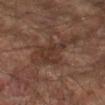Imaged during a routine full-body skin examination; the lesion was not biopsied and no histopathology is available. A roughly 15 mm field-of-view crop from a total-body skin photograph. About 7 mm across. Imaged with cross-polarized lighting. An algorithmic analysis of the crop reported about 7 CIELAB-L* units darker than the surrounding skin and a normalized lesion–skin contrast near 6.5. And it measured a border-irregularity index near 6.5/10, a color-variation rating of about 3/10, and radial color variation of about 1. It also reported an automated nevus-likeness rating near 0 out of 100 and lesion-presence confidence of about 55/100. The lesion is located on the right upper arm. A male patient in their mid- to late 60s.